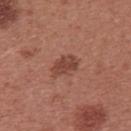{"biopsy_status": "not biopsied; imaged during a skin examination", "image": {"source": "total-body photography crop", "field_of_view_mm": 15}, "lighting": "white-light", "lesion_size": {"long_diameter_mm_approx": 3.5}, "automated_metrics": {"area_mm2_approx": 6.0, "eccentricity": 0.8, "shape_asymmetry": 0.2}, "patient": {"sex": "female", "age_approx": 25}, "site": "upper back"}